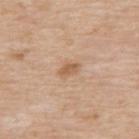Part of a total-body skin-imaging series; this lesion was reviewed on a skin check and was not flagged for biopsy. The subject is a male roughly 80 years of age. The lesion's longest dimension is about 2.5 mm. A 15 mm close-up tile from a total-body photography series done for melanoma screening. Imaged with white-light lighting. On the upper back.